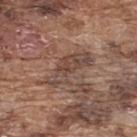Q: Is there a histopathology result?
A: catalogued during a skin exam; not biopsied
Q: What is the anatomic site?
A: the upper back
Q: Illumination type?
A: white-light
Q: Lesion size?
A: ≈5.5 mm
Q: Patient demographics?
A: male, aged around 75
Q: What is the imaging modality?
A: ~15 mm tile from a whole-body skin photo